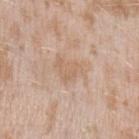workup: no biopsy performed (imaged during a skin exam) | body site: the left upper arm | imaging modality: ~15 mm tile from a whole-body skin photo | subject: female, roughly 25 years of age | tile lighting: white-light.